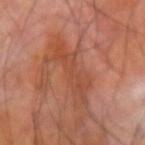• patient: male, about 70 years old
• location: the right forearm
• imaging modality: ~15 mm crop, total-body skin-cancer survey
• TBP lesion metrics: an average lesion color of about L≈47 a*≈26 b*≈32 (CIELAB) and about 6 CIELAB-L* units darker than the surrounding skin; border irregularity of about 8 on a 0–10 scale, internal color variation of about 2 on a 0–10 scale, and radial color variation of about 0.5
• diameter: ~7 mm (longest diameter)
• tile lighting: cross-polarized